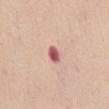This lesion was catalogued during total-body skin photography and was not selected for biopsy. Captured under white-light illumination. A 15 mm crop from a total-body photograph taken for skin-cancer surveillance. From the abdomen. About 2.5 mm across. The subject is a female about 50 years old.